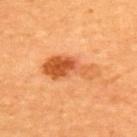Assessment:
This lesion was catalogued during total-body skin photography and was not selected for biopsy.
Context:
Automated tile analysis of the lesion measured a lesion color around L≈59 a*≈31 b*≈46 in CIELAB. It also reported a border-irregularity rating of about 4.5/10, a color-variation rating of about 9.5/10, and peripheral color asymmetry of about 3. The software also gave a nevus-likeness score of about 95/100 and a detector confidence of about 100 out of 100 that the crop contains a lesion. The lesion is located on the upper back. Imaged with cross-polarized lighting. Longest diameter approximately 6.5 mm. A female patient roughly 35 years of age. A 15 mm close-up tile from a total-body photography series done for melanoma screening.